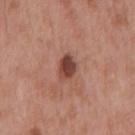Clinical impression: Part of a total-body skin-imaging series; this lesion was reviewed on a skin check and was not flagged for biopsy. Acquisition and patient details: Located on the mid back. Cropped from a whole-body photographic skin survey; the tile spans about 15 mm. Longest diameter approximately 3 mm. Captured under white-light illumination. A male patient, about 55 years old.